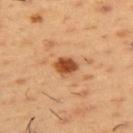Q: Was this lesion biopsied?
A: no biopsy performed (imaged during a skin exam)
Q: What lighting was used for the tile?
A: cross-polarized illumination
Q: Who is the patient?
A: male, aged 53–57
Q: How was this image acquired?
A: ~15 mm tile from a whole-body skin photo
Q: What is the anatomic site?
A: the upper back